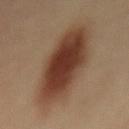Notes:
- biopsy status · total-body-photography surveillance lesion; no biopsy
- acquisition · ~15 mm crop, total-body skin-cancer survey
- diameter · ≈10.5 mm
- illumination · cross-polarized
- subject · female, aged around 35
- anatomic site · the mid back